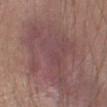biopsy status: no biopsy performed (imaged during a skin exam)
location: the right upper arm
automated lesion analysis: an average lesion color of about L≈46 a*≈20 b*≈17 (CIELAB) and a lesion-to-skin contrast of about 5.5 (normalized; higher = more distinct)
subject: male, aged around 45
image: ~15 mm tile from a whole-body skin photo
size: about 10 mm
illumination: white-light illumination